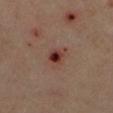Q: Was this lesion biopsied?
A: catalogued during a skin exam; not biopsied
Q: Illumination type?
A: cross-polarized
Q: What are the patient's age and sex?
A: male, aged around 65
Q: Where on the body is the lesion?
A: the left lower leg
Q: How was this image acquired?
A: total-body-photography crop, ~15 mm field of view
Q: How large is the lesion?
A: ≈3 mm